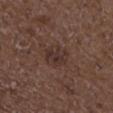{
  "image": {
    "source": "total-body photography crop",
    "field_of_view_mm": 15
  },
  "automated_metrics": {
    "area_mm2_approx": 7.0,
    "eccentricity": 0.4,
    "shape_asymmetry": 0.25,
    "border_irregularity_0_10": 2.5,
    "color_variation_0_10": 3.5,
    "peripheral_color_asymmetry": 1.0,
    "nevus_likeness_0_100": 0,
    "lesion_detection_confidence_0_100": 100
  },
  "patient": {
    "sex": "male",
    "age_approx": 50
  },
  "site": "left forearm",
  "lighting": "white-light"
}